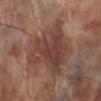Impression:
The lesion was photographed on a routine skin check and not biopsied; there is no pathology result.
Background:
A region of skin cropped from a whole-body photographic capture, roughly 15 mm wide. The recorded lesion diameter is about 8 mm. From the left lower leg. A male patient, in their 70s.